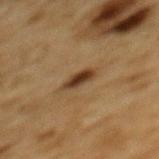Impression:
Imaged during a routine full-body skin examination; the lesion was not biopsied and no histopathology is available.
Image and clinical context:
From the mid back. Longest diameter approximately 3.5 mm. A lesion tile, about 15 mm wide, cut from a 3D total-body photograph. The total-body-photography lesion software estimated a footprint of about 4 mm², an outline eccentricity of about 0.85 (0 = round, 1 = elongated), and a shape-asymmetry score of about 0.3 (0 = symmetric). The software also gave an average lesion color of about L≈33 a*≈16 b*≈28 (CIELAB) and a normalized border contrast of about 11. It also reported an automated nevus-likeness rating near 100 out of 100 and a lesion-detection confidence of about 100/100. A male patient, in their mid-80s or older.